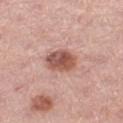Notes:
- patient · female, aged 43 to 47
- size · ~3.5 mm (longest diameter)
- automated metrics · a lesion area of about 8.5 mm² and an outline eccentricity of about 0.5 (0 = round, 1 = elongated); a mean CIELAB color near L≈54 a*≈24 b*≈26, roughly 14 lightness units darker than nearby skin, and a normalized lesion–skin contrast near 9.5; a nevus-likeness score of about 85/100 and a lesion-detection confidence of about 100/100
- image · ~15 mm tile from a whole-body skin photo
- site · the left thigh
- tile lighting · white-light illumination